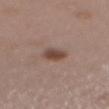Impression:
Recorded during total-body skin imaging; not selected for excision or biopsy.
Background:
About 3 mm across. Imaged with white-light lighting. On the chest. A female subject about 55 years old. A 15 mm close-up tile from a total-body photography series done for melanoma screening.